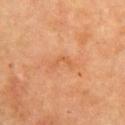notes = total-body-photography surveillance lesion; no biopsy
subject = female, approximately 70 years of age
body site = the right upper arm
lesion diameter = ~3 mm (longest diameter)
image source = total-body-photography crop, ~15 mm field of view
lighting = cross-polarized